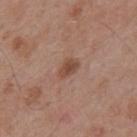* biopsy status: imaged on a skin check; not biopsied
* acquisition: ~15 mm crop, total-body skin-cancer survey
* location: the mid back
* tile lighting: white-light illumination
* size: ~3 mm (longest diameter)
* patient: male, aged approximately 55